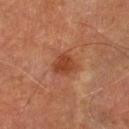Imaged during a routine full-body skin examination; the lesion was not biopsied and no histopathology is available.
Imaged with cross-polarized lighting.
The recorded lesion diameter is about 3.5 mm.
A male subject, aged around 70.
On the leg.
A close-up tile cropped from a whole-body skin photograph, about 15 mm across.